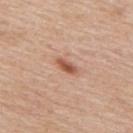Q: Is there a histopathology result?
A: catalogued during a skin exam; not biopsied
Q: How was this image acquired?
A: 15 mm crop, total-body photography
Q: Lesion location?
A: the upper back
Q: Lesion size?
A: about 3 mm
Q: What are the patient's age and sex?
A: male, roughly 60 years of age